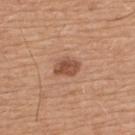Captured during whole-body skin photography for melanoma surveillance; the lesion was not biopsied. The patient is a male aged around 65. From the back. The lesion's longest dimension is about 3 mm. Imaged with white-light lighting. A 15 mm close-up tile from a total-body photography series done for melanoma screening. The total-body-photography lesion software estimated a classifier nevus-likeness of about 65/100.